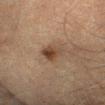The lesion is located on the right forearm. A male patient aged approximately 65. A 15 mm close-up tile from a total-body photography series done for melanoma screening. Longest diameter approximately 4 mm.